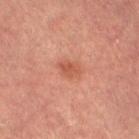The lesion was photographed on a routine skin check and not biopsied; there is no pathology result. Measured at roughly 2.5 mm in maximum diameter. Captured under cross-polarized illumination. This image is a 15 mm lesion crop taken from a total-body photograph. From the left thigh. A female subject, aged around 60.